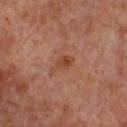biopsy_status: not biopsied; imaged during a skin examination
lesion_size:
  long_diameter_mm_approx: 3.0
image:
  source: total-body photography crop
  field_of_view_mm: 15
automated_metrics:
  area_mm2_approx: 4.0
  eccentricity: 0.85
  shape_asymmetry: 0.4
site: chest
patient:
  sex: female
  age_approx: 60
lighting: cross-polarized